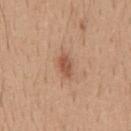The lesion was photographed on a routine skin check and not biopsied; there is no pathology result. From the mid back. This is a white-light tile. Longest diameter approximately 2.5 mm. A close-up tile cropped from a whole-body skin photograph, about 15 mm across. The subject is a male aged around 40.